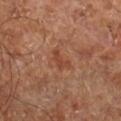| feature | finding |
|---|---|
| patient | in their mid-60s |
| body site | the right lower leg |
| lighting | cross-polarized |
| diameter | ≈3 mm |
| image | 15 mm crop, total-body photography |